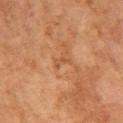* follow-up · catalogued during a skin exam; not biopsied
* image source · 15 mm crop, total-body photography
* lighting · cross-polarized illumination
* subject · female, in their 60s
* location · the left upper arm
* size · ≈2.5 mm